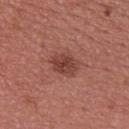Clinical impression: This lesion was catalogued during total-body skin photography and was not selected for biopsy. Clinical summary: Located on the back. A 15 mm close-up extracted from a 3D total-body photography capture. Approximately 3.5 mm at its widest. A male patient aged around 40. The tile uses white-light illumination.